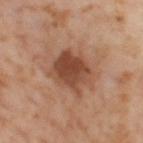Recorded during total-body skin imaging; not selected for excision or biopsy. Cropped from a whole-body photographic skin survey; the tile spans about 15 mm. Located on the left thigh. Measured at roughly 5 mm in maximum diameter. A female subject, aged 53–57. The tile uses cross-polarized illumination.